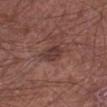Imaged during a routine full-body skin examination; the lesion was not biopsied and no histopathology is available.
A 15 mm crop from a total-body photograph taken for skin-cancer surveillance.
From the left lower leg.
Measured at roughly 3 mm in maximum diameter.
The tile uses white-light illumination.
A male subject, in their mid- to late 60s.